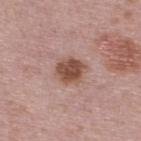{
  "patient": {
    "sex": "male",
    "age_approx": 40
  },
  "site": "upper back",
  "image": {
    "source": "total-body photography crop",
    "field_of_view_mm": 15
  }
}